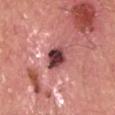follow-up = no biopsy performed (imaged during a skin exam)
acquisition = 15 mm crop, total-body photography
patient = male, in their 60s
anatomic site = the head or neck